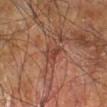Impression: Part of a total-body skin-imaging series; this lesion was reviewed on a skin check and was not flagged for biopsy. Context: Measured at roughly 3 mm in maximum diameter. Captured under cross-polarized illumination. On the right leg. The patient is a male aged 58 to 62. Automated tile analysis of the lesion measured a footprint of about 3.5 mm², a shape eccentricity near 0.85, and a shape-asymmetry score of about 0.35 (0 = symmetric). A 15 mm close-up tile from a total-body photography series done for melanoma screening.